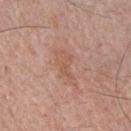follow-up = no biopsy performed (imaged during a skin exam)
subject = male, approximately 50 years of age
imaging modality = ~15 mm crop, total-body skin-cancer survey
location = the chest
lighting = white-light illumination
automated lesion analysis = an area of roughly 7.5 mm²; a lesion color around L≈57 a*≈21 b*≈29 in CIELAB, a lesion–skin lightness drop of about 6, and a normalized lesion–skin contrast near 5